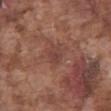biopsy status: imaged on a skin check; not biopsied
patient: male, approximately 75 years of age
automated lesion analysis: a lesion area of about 4 mm², an outline eccentricity of about 0.6 (0 = round, 1 = elongated), and two-axis asymmetry of about 0.2; a border-irregularity index near 1.5/10 and a within-lesion color-variation index near 2/10
image source: total-body-photography crop, ~15 mm field of view
diameter: ~2.5 mm (longest diameter)
anatomic site: the abdomen
illumination: white-light illumination